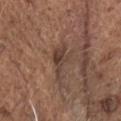workup: no biopsy performed (imaged during a skin exam); illumination: white-light; image: ~15 mm crop, total-body skin-cancer survey; lesion size: ≈4.5 mm; location: the head or neck; subject: male, in their mid- to late 70s; automated metrics: an average lesion color of about L≈40 a*≈17 b*≈24 (CIELAB), a lesion–skin lightness drop of about 8, and a normalized border contrast of about 7.